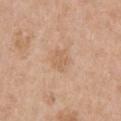biopsy_status: not biopsied; imaged during a skin examination
patient:
  sex: male
  age_approx: 65
automated_metrics:
  cielab_L: 62
  cielab_a: 18
  cielab_b: 34
  vs_skin_darker_L: 6.0
  vs_skin_contrast_norm: 4.5
  color_variation_0_10: 1.5
  peripheral_color_asymmetry: 0.5
site: chest
image:
  source: total-body photography crop
  field_of_view_mm: 15
lesion_size:
  long_diameter_mm_approx: 2.5